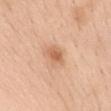* biopsy status · imaged on a skin check; not biopsied
* diameter · about 2.5 mm
* body site · the chest
* patient · female, aged approximately 55
* acquisition · total-body-photography crop, ~15 mm field of view
* illumination · white-light illumination